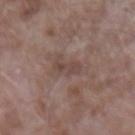follow-up: catalogued during a skin exam; not biopsied | diameter: about 3 mm | patient: male, in their mid-60s | image source: ~15 mm crop, total-body skin-cancer survey | TBP lesion metrics: a lesion–skin lightness drop of about 7; a border-irregularity index near 4.5/10, internal color variation of about 0.5 on a 0–10 scale, and peripheral color asymmetry of about 0 | tile lighting: white-light | location: the left forearm.